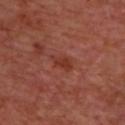Q: Was a biopsy performed?
A: total-body-photography surveillance lesion; no biopsy
Q: Automated lesion metrics?
A: an area of roughly 5 mm², a shape eccentricity near 0.8, and two-axis asymmetry of about 0.25; an average lesion color of about L≈35 a*≈27 b*≈28 (CIELAB) and a lesion–skin lightness drop of about 6; a border-irregularity rating of about 3/10 and internal color variation of about 2.5 on a 0–10 scale; a classifier nevus-likeness of about 10/100 and a lesion-detection confidence of about 100/100
Q: How was the tile lit?
A: cross-polarized illumination
Q: How was this image acquired?
A: ~15 mm crop, total-body skin-cancer survey
Q: What is the anatomic site?
A: the upper back
Q: What is the lesion's diameter?
A: ≈3.5 mm
Q: What are the patient's age and sex?
A: male, about 65 years old